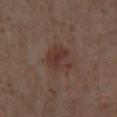Impression: Captured during whole-body skin photography for melanoma surveillance; the lesion was not biopsied. Context: Captured under cross-polarized illumination. The lesion's longest dimension is about 3.5 mm. The total-body-photography lesion software estimated a footprint of about 7.5 mm² and an eccentricity of roughly 0.5. And it measured a border-irregularity rating of about 3.5/10, internal color variation of about 5 on a 0–10 scale, and radial color variation of about 2. The analysis additionally found a lesion-detection confidence of about 100/100. A female subject roughly 55 years of age. On the left lower leg. Cropped from a total-body skin-imaging series; the visible field is about 15 mm.